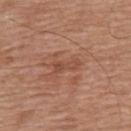Impression:
Captured during whole-body skin photography for melanoma surveillance; the lesion was not biopsied.
Clinical summary:
A lesion tile, about 15 mm wide, cut from a 3D total-body photograph. Approximately 3 mm at its widest. A male patient about 65 years old. Located on the mid back. This is a white-light tile.